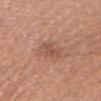notes = catalogued during a skin exam; not biopsied.